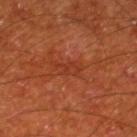patient:
  sex: male
  age_approx: 65
image:
  source: total-body photography crop
  field_of_view_mm: 15
lesion_size:
  long_diameter_mm_approx: 3.5
site: right lower leg